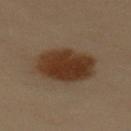  biopsy_status: not biopsied; imaged during a skin examination
  image:
    source: total-body photography crop
    field_of_view_mm: 15
  automated_metrics:
    area_mm2_approx: 23.0
    eccentricity: 0.8
    shape_asymmetry: 0.1
  site: back
  patient:
    sex: female
    age_approx: 60